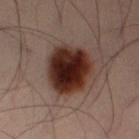follow-up: imaged on a skin check; not biopsied | anatomic site: the leg | imaging modality: total-body-photography crop, ~15 mm field of view | patient: male, roughly 40 years of age | tile lighting: cross-polarized illumination | diameter: ~6 mm (longest diameter) | TBP lesion metrics: a border-irregularity index near 1.5/10, internal color variation of about 7 on a 0–10 scale, and peripheral color asymmetry of about 2.